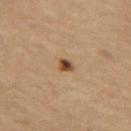Case summary:
- workup · no biopsy performed (imaged during a skin exam)
- patient · female, aged 58–62
- size · ~2 mm (longest diameter)
- site · the leg
- image-analysis metrics · roughly 15 lightness units darker than nearby skin and a normalized border contrast of about 11; a border-irregularity index near 2/10, a within-lesion color-variation index near 5/10, and peripheral color asymmetry of about 1.5; a classifier nevus-likeness of about 100/100 and a lesion-detection confidence of about 100/100
- acquisition · 15 mm crop, total-body photography
- illumination · cross-polarized illumination